This image is a 15 mm lesion crop taken from a total-body photograph; this is a white-light tile; about 1 mm across; the patient is a male aged around 55; The total-body-photography lesion software estimated an area of roughly 1 mm², an eccentricity of roughly 0.8, and a shape-asymmetry score of about 0.5 (0 = symmetric). The software also gave an average lesion color of about L≈44 a*≈34 b*≈25 (CIELAB) and a normalized lesion–skin contrast near 3.5. The analysis additionally found an automated nevus-likeness rating near 0 out of 100; the lesion is on the front of the torso:
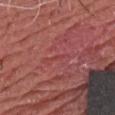  diagnosis:
    histopathology: squamous cell carcinoma in situ
    malignancy: malignant
    taxonomic_path:
      - Malignant
      - Malignant epidermal proliferations
      - Squamous cell carcinoma in situ